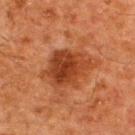This lesion was catalogued during total-body skin photography and was not selected for biopsy. A male patient aged 58–62. The tile uses cross-polarized illumination. Cropped from a total-body skin-imaging series; the visible field is about 15 mm. The lesion-visualizer software estimated an area of roughly 16 mm², an eccentricity of roughly 0.65, and two-axis asymmetry of about 0.25. Located on the upper back.